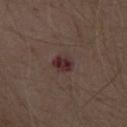Assessment:
This lesion was catalogued during total-body skin photography and was not selected for biopsy.
Background:
From the abdomen. A roughly 15 mm field-of-view crop from a total-body skin photograph. Approximately 2.5 mm at its widest. The subject is a male aged 68 to 72.